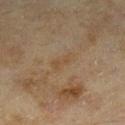{
  "biopsy_status": "not biopsied; imaged during a skin examination",
  "lighting": "cross-polarized",
  "image": {
    "source": "total-body photography crop",
    "field_of_view_mm": 15
  },
  "lesion_size": {
    "long_diameter_mm_approx": 3.5
  },
  "site": "right lower leg",
  "patient": {
    "sex": "female",
    "age_approx": 60
  }
}